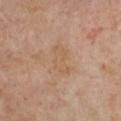Case summary:
- workup: no biopsy performed (imaged during a skin exam)
- tile lighting: cross-polarized illumination
- lesion size: ≈4 mm
- body site: the chest
- subject: female, roughly 60 years of age
- acquisition: ~15 mm crop, total-body skin-cancer survey